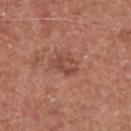Q: Is there a histopathology result?
A: catalogued during a skin exam; not biopsied
Q: What lighting was used for the tile?
A: white-light
Q: Lesion size?
A: ~3 mm (longest diameter)
Q: What is the imaging modality?
A: 15 mm crop, total-body photography
Q: What did automated image analysis measure?
A: a footprint of about 5 mm²; a color-variation rating of about 4/10 and radial color variation of about 1.5; a detector confidence of about 100 out of 100 that the crop contains a lesion
Q: Patient demographics?
A: male, aged approximately 65
Q: Where on the body is the lesion?
A: the upper back